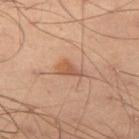This lesion was catalogued during total-body skin photography and was not selected for biopsy. Measured at roughly 3.5 mm in maximum diameter. A male patient aged 53 to 57. On the left thigh. The lesion-visualizer software estimated a border-irregularity rating of about 3.5/10, internal color variation of about 2 on a 0–10 scale, and radial color variation of about 0.5. It also reported a lesion-detection confidence of about 100/100. Imaged with cross-polarized lighting. This image is a 15 mm lesion crop taken from a total-body photograph.